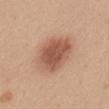Impression:
No biopsy was performed on this lesion — it was imaged during a full skin examination and was not determined to be concerning.
Acquisition and patient details:
A close-up tile cropped from a whole-body skin photograph, about 15 mm across. The patient is a female roughly 45 years of age. On the back. The tile uses white-light illumination. Longest diameter approximately 6 mm.